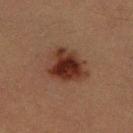<case>
<biopsy_status>not biopsied; imaged during a skin examination</biopsy_status>
<lesion_size>
  <long_diameter_mm_approx>5.0</long_diameter_mm_approx>
</lesion_size>
<patient>
  <sex>male</sex>
  <age_approx>40</age_approx>
</patient>
<image>
  <source>total-body photography crop</source>
  <field_of_view_mm>15</field_of_view_mm>
</image>
<automated_metrics>
  <border_irregularity_0_10>2.5</border_irregularity_0_10>
  <color_variation_0_10>5.5</color_variation_0_10>
  <peripheral_color_asymmetry>1.5</peripheral_color_asymmetry>
  <lesion_detection_confidence_0_100>100</lesion_detection_confidence_0_100>
</automated_metrics>
<site>left thigh</site>
<lighting>cross-polarized</lighting>
</case>